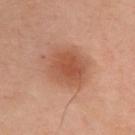The lesion was photographed on a routine skin check and not biopsied; there is no pathology result. A close-up tile cropped from a whole-body skin photograph, about 15 mm across. This is a cross-polarized tile. The lesion-visualizer software estimated an area of roughly 17 mm² and a symmetry-axis asymmetry near 0.15. It also reported a mean CIELAB color near L≈54 a*≈25 b*≈33 and a normalized lesion–skin contrast near 7. And it measured an automated nevus-likeness rating near 95 out of 100 and a lesion-detection confidence of about 100/100. From the right upper arm. A female subject, about 55 years old.